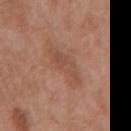Captured during whole-body skin photography for melanoma surveillance; the lesion was not biopsied. The patient is a male in their mid- to late 70s. A lesion tile, about 15 mm wide, cut from a 3D total-body photograph. The lesion is on the mid back. An algorithmic analysis of the crop reported a classifier nevus-likeness of about 0/100. Approximately 5 mm at its widest.